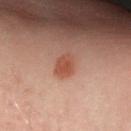follow-up: catalogued during a skin exam; not biopsied
body site: the left arm
automated lesion analysis: a lesion area of about 4.5 mm², an outline eccentricity of about 0.65 (0 = round, 1 = elongated), and a shape-asymmetry score of about 0.2 (0 = symmetric); a mean CIELAB color near L≈40 a*≈22 b*≈26, roughly 7 lightness units darker than nearby skin, and a normalized border contrast of about 7; internal color variation of about 2.5 on a 0–10 scale and peripheral color asymmetry of about 1; an automated nevus-likeness rating near 100 out of 100 and lesion-presence confidence of about 100/100
illumination: cross-polarized
subject: male, aged 48–52
imaging modality: 15 mm crop, total-body photography